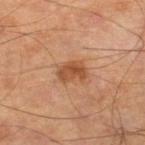Clinical impression: The lesion was tiled from a total-body skin photograph and was not biopsied.